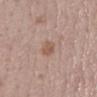On the mid back.
Measured at roughly 3 mm in maximum diameter.
A lesion tile, about 15 mm wide, cut from a 3D total-body photograph.
A male subject, aged around 70.